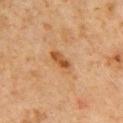Part of a total-body skin-imaging series; this lesion was reviewed on a skin check and was not flagged for biopsy.
The lesion is on the chest.
This is a cross-polarized tile.
The total-body-photography lesion software estimated about 9 CIELAB-L* units darker than the surrounding skin and a lesion-to-skin contrast of about 8 (normalized; higher = more distinct). The analysis additionally found a border-irregularity index near 2.5/10.
Measured at roughly 3.5 mm in maximum diameter.
A 15 mm crop from a total-body photograph taken for skin-cancer surveillance.
A male subject, approximately 60 years of age.